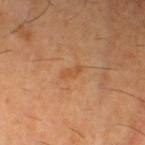Clinical impression: The lesion was photographed on a routine skin check and not biopsied; there is no pathology result. Background: An algorithmic analysis of the crop reported an average lesion color of about L≈49 a*≈22 b*≈37 (CIELAB) and a normalized border contrast of about 5. It also reported a border-irregularity rating of about 4/10, a within-lesion color-variation index near 0/10, and radial color variation of about 0. A close-up tile cropped from a whole-body skin photograph, about 15 mm across. Located on the left thigh. Imaged with cross-polarized lighting. A male subject, aged 58 to 62.